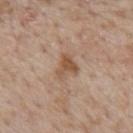Q: Was this lesion biopsied?
A: imaged on a skin check; not biopsied